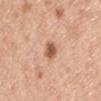  biopsy_status: not biopsied; imaged during a skin examination
  lesion_size:
    long_diameter_mm_approx: 2.5
  lighting: white-light
  image:
    source: total-body photography crop
    field_of_view_mm: 15
  site: left upper arm
  automated_metrics:
    area_mm2_approx: 4.0
    eccentricity: 0.6
    shape_asymmetry: 0.2
    vs_skin_darker_L: 14.0
    vs_skin_contrast_norm: 9.0
    border_irregularity_0_10: 2.0
  patient:
    sex: male
    age_approx: 40